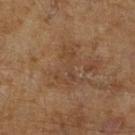Captured during whole-body skin photography for melanoma surveillance; the lesion was not biopsied.
A lesion tile, about 15 mm wide, cut from a 3D total-body photograph.
Longest diameter approximately 4.5 mm.
Captured under cross-polarized illumination.
The subject is a male aged 63–67.